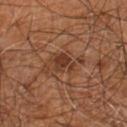Clinical impression: The lesion was photographed on a routine skin check and not biopsied; there is no pathology result. Image and clinical context: A roughly 15 mm field-of-view crop from a total-body skin photograph. A male patient in their 60s. From the right leg.